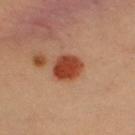<lesion>
  <biopsy_status>not biopsied; imaged during a skin examination</biopsy_status>
</lesion>